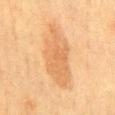{"biopsy_status": "not biopsied; imaged during a skin examination", "site": "mid back", "patient": {"sex": "female", "age_approx": 55}, "automated_metrics": {"cielab_L": 57, "cielab_a": 20, "cielab_b": 37, "vs_skin_darker_L": 8.0, "vs_skin_contrast_norm": 6.0, "border_irregularity_0_10": 4.5, "color_variation_0_10": 2.5}, "image": {"source": "total-body photography crop", "field_of_view_mm": 15}, "lesion_size": {"long_diameter_mm_approx": 9.5}}